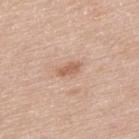| feature | finding |
|---|---|
| notes | imaged on a skin check; not biopsied |
| illumination | white-light illumination |
| image source | ~15 mm crop, total-body skin-cancer survey |
| anatomic site | the back |
| patient | male, aged 63–67 |